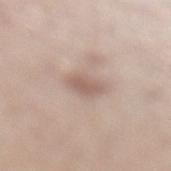Part of a total-body skin-imaging series; this lesion was reviewed on a skin check and was not flagged for biopsy.
The tile uses white-light illumination.
A roughly 15 mm field-of-view crop from a total-body skin photograph.
From the left lower leg.
The subject is a female in their mid-50s.